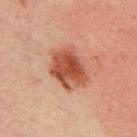<case>
  <biopsy_status>not biopsied; imaged during a skin examination</biopsy_status>
  <image>
    <source>total-body photography crop</source>
    <field_of_view_mm>15</field_of_view_mm>
  </image>
  <site>mid back</site>
  <patient>
    <sex>male</sex>
    <age_approx>30</age_approx>
  </patient>
</case>